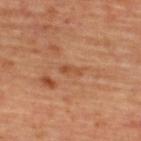A female patient, in their mid- to late 40s. The lesion's longest dimension is about 2.5 mm. From the upper back. A region of skin cropped from a whole-body photographic capture, roughly 15 mm wide.